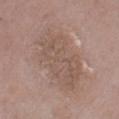Q: Was a biopsy performed?
A: no biopsy performed (imaged during a skin exam)
Q: How large is the lesion?
A: ≈9 mm
Q: What kind of image is this?
A: total-body-photography crop, ~15 mm field of view
Q: What is the anatomic site?
A: the right thigh
Q: Automated lesion metrics?
A: a border-irregularity rating of about 3/10, a within-lesion color-variation index near 3.5/10, and peripheral color asymmetry of about 1
Q: Who is the patient?
A: male, in their mid- to late 70s
Q: Illumination type?
A: white-light